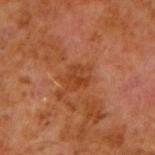Recorded during total-body skin imaging; not selected for excision or biopsy. A male subject aged approximately 60. From the right upper arm. Measured at roughly 3.5 mm in maximum diameter. A 15 mm close-up tile from a total-body photography series done for melanoma screening.